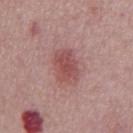Assessment: Recorded during total-body skin imaging; not selected for excision or biopsy. Acquisition and patient details: A roughly 15 mm field-of-view crop from a total-body skin photograph. Captured under white-light illumination. Measured at roughly 4 mm in maximum diameter. The subject is a male aged 73 to 77. The lesion is located on the abdomen. The lesion-visualizer software estimated an area of roughly 10 mm², a shape eccentricity near 0.7, and two-axis asymmetry of about 0.15. It also reported an automated nevus-likeness rating near 75 out of 100 and a lesion-detection confidence of about 100/100.